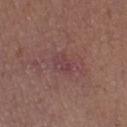notes — no biopsy performed (imaged during a skin exam) | imaging modality — ~15 mm tile from a whole-body skin photo | location — the right lower leg | automated metrics — an outline eccentricity of about 0.8 (0 = round, 1 = elongated); a nevus-likeness score of about 0/100 | patient — female, aged 38–42 | illumination — white-light.